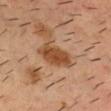The lesion was photographed on a routine skin check and not biopsied; there is no pathology result.
A close-up tile cropped from a whole-body skin photograph, about 15 mm across.
This is a cross-polarized tile.
Located on the head or neck.
Automated tile analysis of the lesion measured a lesion area of about 11 mm², a shape eccentricity near 0.7, and a shape-asymmetry score of about 0.2 (0 = symmetric). The software also gave a border-irregularity rating of about 2.5/10 and a within-lesion color-variation index near 3/10.
A male patient, aged 33 to 37.